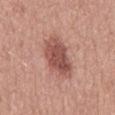Q: Was this lesion biopsied?
A: catalogued during a skin exam; not biopsied
Q: How was the tile lit?
A: white-light illumination
Q: Lesion size?
A: ~5.5 mm (longest diameter)
Q: What is the imaging modality?
A: ~15 mm tile from a whole-body skin photo
Q: What are the patient's age and sex?
A: male, about 50 years old
Q: Lesion location?
A: the back